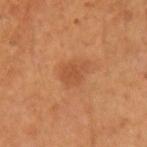The lesion was tiled from a total-body skin photograph and was not biopsied. A 15 mm close-up extracted from a 3D total-body photography capture. The tile uses cross-polarized illumination. The lesion-visualizer software estimated an automated nevus-likeness rating near 20 out of 100 and a lesion-detection confidence of about 100/100. The subject is a female about 45 years old. Located on the right forearm. Approximately 3.5 mm at its widest.